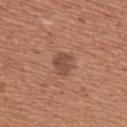This lesion was catalogued during total-body skin photography and was not selected for biopsy. The lesion is on the back. The recorded lesion diameter is about 2.5 mm. A lesion tile, about 15 mm wide, cut from a 3D total-body photograph. A female subject, aged approximately 50.